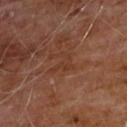Recorded during total-body skin imaging; not selected for excision or biopsy. Located on the chest. The subject is a male aged 58–62. Measured at roughly 3.5 mm in maximum diameter. A close-up tile cropped from a whole-body skin photograph, about 15 mm across.